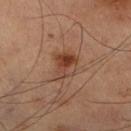Impression: The lesion was photographed on a routine skin check and not biopsied; there is no pathology result. Image and clinical context: Located on the right lower leg. A 15 mm close-up extracted from a 3D total-body photography capture. The total-body-photography lesion software estimated an outline eccentricity of about 0.7 (0 = round, 1 = elongated) and a symmetry-axis asymmetry near 0.3. The software also gave a border-irregularity index near 3/10, internal color variation of about 9 on a 0–10 scale, and peripheral color asymmetry of about 3. The analysis additionally found a classifier nevus-likeness of about 90/100 and lesion-presence confidence of about 100/100. Approximately 4 mm at its widest. The patient is a male roughly 60 years of age.